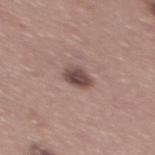Assessment:
Imaged during a routine full-body skin examination; the lesion was not biopsied and no histopathology is available.
Clinical summary:
Located on the mid back. The patient is a male aged approximately 40. The tile uses white-light illumination. A 15 mm close-up extracted from a 3D total-body photography capture. An algorithmic analysis of the crop reported a symmetry-axis asymmetry near 0.2. And it measured border irregularity of about 1.5 on a 0–10 scale and peripheral color asymmetry of about 1. And it measured a classifier nevus-likeness of about 50/100.